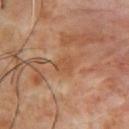Case summary:
• workup — total-body-photography surveillance lesion; no biopsy
• body site — the front of the torso
• subject — male, aged 53–57
• lesion size — ~2.5 mm (longest diameter)
• image — total-body-photography crop, ~15 mm field of view
• tile lighting — cross-polarized illumination